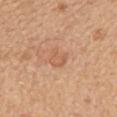Part of a total-body skin-imaging series; this lesion was reviewed on a skin check and was not flagged for biopsy. The recorded lesion diameter is about 2.5 mm. A region of skin cropped from a whole-body photographic capture, roughly 15 mm wide. From the mid back. A female patient roughly 55 years of age.